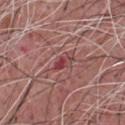The lesion was photographed on a routine skin check and not biopsied; there is no pathology result.
Located on the chest.
Longest diameter approximately 2.5 mm.
An algorithmic analysis of the crop reported a footprint of about 3 mm² and an outline eccentricity of about 0.85 (0 = round, 1 = elongated). The analysis additionally found a lesion color around L≈42 a*≈30 b*≈20 in CIELAB. The analysis additionally found internal color variation of about 2 on a 0–10 scale and a peripheral color-asymmetry measure near 0.5.
Imaged with white-light lighting.
A male subject, aged 53–57.
Cropped from a whole-body photographic skin survey; the tile spans about 15 mm.